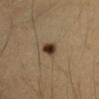Clinical impression: This lesion was catalogued during total-body skin photography and was not selected for biopsy. Context: The lesion's longest dimension is about 2.5 mm. Located on the arm. Captured under cross-polarized illumination. A female subject, aged approximately 35. Cropped from a total-body skin-imaging series; the visible field is about 15 mm.